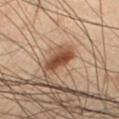Captured during whole-body skin photography for melanoma surveillance; the lesion was not biopsied. Automated tile analysis of the lesion measured an area of roughly 6 mm², a shape eccentricity near 0.75, and two-axis asymmetry of about 0.25. And it measured a lesion color around L≈37 a*≈18 b*≈26 in CIELAB, about 12 CIELAB-L* units darker than the surrounding skin, and a normalized border contrast of about 10.5. And it measured a border-irregularity rating of about 2.5/10, a within-lesion color-variation index near 2.5/10, and a peripheral color-asymmetry measure near 1. The software also gave a nevus-likeness score of about 95/100. A lesion tile, about 15 mm wide, cut from a 3D total-body photograph. The subject is a male aged 53–57. Imaged with cross-polarized lighting. On the left lower leg. Longest diameter approximately 3 mm.